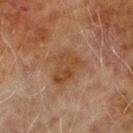Image and clinical context:
Longest diameter approximately 4.5 mm. From the arm. A 15 mm close-up tile from a total-body photography series done for melanoma screening. Imaged with cross-polarized lighting. The total-body-photography lesion software estimated a border-irregularity index near 4.5/10, internal color variation of about 3.5 on a 0–10 scale, and peripheral color asymmetry of about 1. The subject is a male aged 68 to 72.